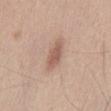notes = no biopsy performed (imaged during a skin exam)
automated metrics = a normalized lesion–skin contrast near 7; a color-variation rating of about 2/10 and a peripheral color-asymmetry measure near 0.5
anatomic site = the abdomen
imaging modality = ~15 mm crop, total-body skin-cancer survey
subject = male, in their mid-40s
size = ≈4 mm